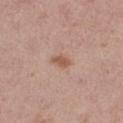Q: Is there a histopathology result?
A: imaged on a skin check; not biopsied
Q: How was this image acquired?
A: ~15 mm crop, total-body skin-cancer survey
Q: Patient demographics?
A: female, aged 58 to 62
Q: What did automated image analysis measure?
A: a footprint of about 3 mm² and an outline eccentricity of about 0.85 (0 = round, 1 = elongated); a mean CIELAB color near L≈55 a*≈21 b*≈29, about 9 CIELAB-L* units darker than the surrounding skin, and a normalized border contrast of about 7
Q: Lesion size?
A: ~2.5 mm (longest diameter)
Q: How was the tile lit?
A: white-light illumination
Q: Where on the body is the lesion?
A: the right thigh